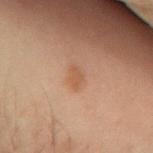biopsy status — catalogued during a skin exam; not biopsied
location — the right forearm
image — ~15 mm tile from a whole-body skin photo
lesion size — ≈2.5 mm
patient — male, aged around 50
tile lighting — cross-polarized
automated metrics — a footprint of about 3.5 mm², an eccentricity of roughly 0.75, and a shape-asymmetry score of about 0.25 (0 = symmetric); a lesion color around L≈42 a*≈17 b*≈27 in CIELAB, a lesion–skin lightness drop of about 5, and a lesion-to-skin contrast of about 5.5 (normalized; higher = more distinct); a border-irregularity index near 2/10, internal color variation of about 1 on a 0–10 scale, and peripheral color asymmetry of about 0.5